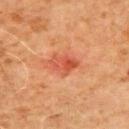Clinical impression:
Part of a total-body skin-imaging series; this lesion was reviewed on a skin check and was not flagged for biopsy.
Acquisition and patient details:
A 15 mm close-up tile from a total-body photography series done for melanoma screening. A male subject aged 68–72. From the left upper arm.